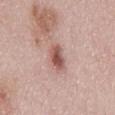<tbp_lesion>
  <biopsy_status>not biopsied; imaged during a skin examination</biopsy_status>
  <image>
    <source>total-body photography crop</source>
    <field_of_view_mm>15</field_of_view_mm>
  </image>
  <site>mid back</site>
  <patient>
    <sex>female</sex>
    <age_approx>30</age_approx>
  </patient>
</tbp_lesion>